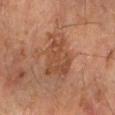The lesion was tiled from a total-body skin photograph and was not biopsied.